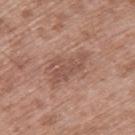Recorded during total-body skin imaging; not selected for excision or biopsy. A male patient, approximately 50 years of age. An algorithmic analysis of the crop reported an average lesion color of about L≈52 a*≈21 b*≈26 (CIELAB), about 8 CIELAB-L* units darker than the surrounding skin, and a normalized border contrast of about 6. The software also gave a border-irregularity index near 6/10, internal color variation of about 2 on a 0–10 scale, and radial color variation of about 0.5. A 15 mm close-up tile from a total-body photography series done for melanoma screening. Longest diameter approximately 5 mm. Located on the upper back.